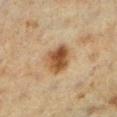This lesion was catalogued during total-body skin photography and was not selected for biopsy. This is a cross-polarized tile. A female subject approximately 55 years of age. Approximately 3.5 mm at its widest. A lesion tile, about 15 mm wide, cut from a 3D total-body photograph. From the left lower leg.